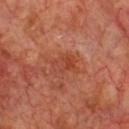The lesion was tiled from a total-body skin photograph and was not biopsied.
Measured at roughly 3.5 mm in maximum diameter.
The lesion is on the chest.
A lesion tile, about 15 mm wide, cut from a 3D total-body photograph.
The subject is a male aged 68–72.
Captured under cross-polarized illumination.